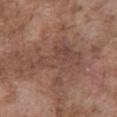automated_metrics:
  nevus_likeness_0_100: 0
  lesion_detection_confidence_0_100: 50
patient:
  sex: male
  age_approx: 75
lighting: white-light
site: abdomen
lesion_size:
  long_diameter_mm_approx: 7.5
image:
  source: total-body photography crop
  field_of_view_mm: 15